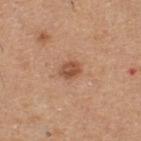Part of a total-body skin-imaging series; this lesion was reviewed on a skin check and was not flagged for biopsy.
The lesion's longest dimension is about 2.5 mm.
This is a white-light tile.
A male patient aged 58 to 62.
Cropped from a total-body skin-imaging series; the visible field is about 15 mm.
Located on the upper back.
The total-body-photography lesion software estimated a mean CIELAB color near L≈52 a*≈23 b*≈33, roughly 11 lightness units darker than nearby skin, and a normalized lesion–skin contrast near 8. And it measured a classifier nevus-likeness of about 85/100 and a lesion-detection confidence of about 100/100.